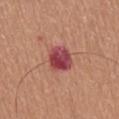{"image": {"source": "total-body photography crop", "field_of_view_mm": 15}, "lesion_size": {"long_diameter_mm_approx": 3.0}, "automated_metrics": {"area_mm2_approx": 9.0, "eccentricity": 0.3, "shape_asymmetry": 0.2, "vs_skin_contrast_norm": 11.0}, "site": "lower back", "patient": {"sex": "male", "age_approx": 60}, "lighting": "white-light"}